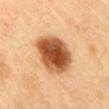| field | value |
|---|---|
| notes | imaged on a skin check; not biopsied |
| body site | the mid back |
| image source | total-body-photography crop, ~15 mm field of view |
| automated metrics | a mean CIELAB color near L≈56 a*≈26 b*≈41, a lesion–skin lightness drop of about 21, and a normalized lesion–skin contrast near 12.5 |
| subject | female, aged approximately 60 |
| lesion diameter | ~6 mm (longest diameter) |